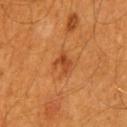Part of a total-body skin-imaging series; this lesion was reviewed on a skin check and was not flagged for biopsy. On the back. Automated tile analysis of the lesion measured an area of roughly 4 mm², an eccentricity of roughly 0.65, and a shape-asymmetry score of about 0.4 (0 = symmetric). The analysis additionally found a lesion color around L≈44 a*≈28 b*≈40 in CIELAB, roughly 8 lightness units darker than nearby skin, and a normalized border contrast of about 6.5. The software also gave a nevus-likeness score of about 70/100 and a lesion-detection confidence of about 100/100. A male patient roughly 60 years of age. A lesion tile, about 15 mm wide, cut from a 3D total-body photograph. Imaged with cross-polarized lighting. The recorded lesion diameter is about 2.5 mm.